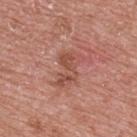| key | value |
|---|---|
| workup | total-body-photography surveillance lesion; no biopsy |
| acquisition | ~15 mm crop, total-body skin-cancer survey |
| body site | the upper back |
| patient | male, aged approximately 70 |